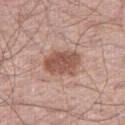Part of a total-body skin-imaging series; this lesion was reviewed on a skin check and was not flagged for biopsy.
A close-up tile cropped from a whole-body skin photograph, about 15 mm across.
Automated tile analysis of the lesion measured a lesion area of about 12 mm², an eccentricity of roughly 0.7, and two-axis asymmetry of about 0.2. The software also gave a border-irregularity rating of about 2/10, internal color variation of about 3.5 on a 0–10 scale, and a peripheral color-asymmetry measure near 1.5. It also reported an automated nevus-likeness rating near 95 out of 100 and lesion-presence confidence of about 100/100.
A male subject, aged around 60.
On the right thigh.